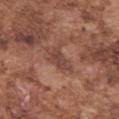biopsy status: imaged on a skin check; not biopsied | body site: the left upper arm | illumination: white-light illumination | lesion diameter: ≈4.5 mm | patient: male, roughly 75 years of age | image: 15 mm crop, total-body photography.